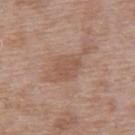Assessment:
This lesion was catalogued during total-body skin photography and was not selected for biopsy.
Acquisition and patient details:
This is a white-light tile. A close-up tile cropped from a whole-body skin photograph, about 15 mm across. A male patient, aged approximately 50. Measured at roughly 3 mm in maximum diameter. On the upper back.